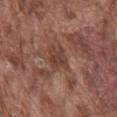The lesion was tiled from a total-body skin photograph and was not biopsied. Automated tile analysis of the lesion measured a footprint of about 6 mm² and two-axis asymmetry of about 0.25. The analysis additionally found a mean CIELAB color near L≈40 a*≈20 b*≈24 and roughly 8 lightness units darker than nearby skin. The analysis additionally found a border-irregularity index near 3/10, internal color variation of about 2 on a 0–10 scale, and radial color variation of about 1. From the mid back. Imaged with white-light lighting. A close-up tile cropped from a whole-body skin photograph, about 15 mm across. A male subject aged approximately 75.